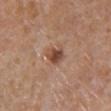Impression: Captured during whole-body skin photography for melanoma surveillance; the lesion was not biopsied. Acquisition and patient details: Measured at roughly 3 mm in maximum diameter. Automated tile analysis of the lesion measured an average lesion color of about L≈46 a*≈21 b*≈29 (CIELAB) and a normalized lesion–skin contrast near 9. The analysis additionally found a border-irregularity index near 2.5/10 and peripheral color asymmetry of about 1.5. The analysis additionally found a nevus-likeness score of about 90/100 and a detector confidence of about 100 out of 100 that the crop contains a lesion. On the left lower leg. The subject is a female aged 53–57. Cropped from a total-body skin-imaging series; the visible field is about 15 mm.